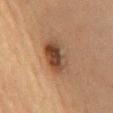Clinical impression:
Part of a total-body skin-imaging series; this lesion was reviewed on a skin check and was not flagged for biopsy.
Context:
A 15 mm crop from a total-body photograph taken for skin-cancer surveillance. On the chest. The subject is a female aged approximately 60.